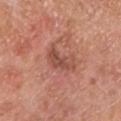Clinical impression: No biopsy was performed on this lesion — it was imaged during a full skin examination and was not determined to be concerning. Image and clinical context: A region of skin cropped from a whole-body photographic capture, roughly 15 mm wide. A male subject roughly 80 years of age. From the leg. The tile uses white-light illumination. Longest diameter approximately 4.5 mm.